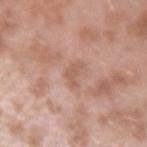  site: left forearm
  automated_metrics:
    cielab_L: 57
    cielab_a: 21
    cielab_b: 29
    vs_skin_darker_L: 7.0
    nevus_likeness_0_100: 0
    lesion_detection_confidence_0_100: 100
  patient:
    sex: male
    age_approx: 40
  lighting: white-light
  lesion_size:
    long_diameter_mm_approx: 2.5
  image:
    source: total-body photography crop
    field_of_view_mm: 15